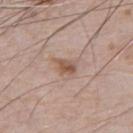Case summary:
* image-analysis metrics · a lesion area of about 4 mm², a shape eccentricity near 0.75, and two-axis asymmetry of about 0.3; an average lesion color of about L≈54 a*≈18 b*≈27 (CIELAB) and roughly 10 lightness units darker than nearby skin
* tile lighting · white-light illumination
* acquisition · total-body-photography crop, ~15 mm field of view
* location · the abdomen
* size · about 3 mm
* patient · male, in their 80s